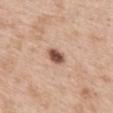Captured during whole-body skin photography for melanoma surveillance; the lesion was not biopsied. The lesion is on the back. A close-up tile cropped from a whole-body skin photograph, about 15 mm across. A female subject, roughly 40 years of age. This is a white-light tile. About 3 mm across.